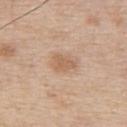biopsy status: no biopsy performed (imaged during a skin exam) | automated metrics: a lesion area of about 5 mm², an eccentricity of roughly 0.75, and two-axis asymmetry of about 0.2; an average lesion color of about L≈61 a*≈18 b*≈32 (CIELAB), roughly 8 lightness units darker than nearby skin, and a normalized lesion–skin contrast near 6; border irregularity of about 2 on a 0–10 scale, a within-lesion color-variation index near 1.5/10, and peripheral color asymmetry of about 0.5; an automated nevus-likeness rating near 5 out of 100 | size: ~3 mm (longest diameter) | illumination: white-light illumination | patient: male, roughly 55 years of age | image: 15 mm crop, total-body photography | location: the upper back.